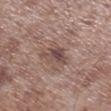Clinical impression:
The lesion was tiled from a total-body skin photograph and was not biopsied.
Context:
A 15 mm close-up tile from a total-body photography series done for melanoma screening. Imaged with white-light lighting. From the right lower leg. Longest diameter approximately 3.5 mm. A male subject, aged 53–57. An algorithmic analysis of the crop reported a footprint of about 5.5 mm², an eccentricity of roughly 0.75, and a symmetry-axis asymmetry near 0.4. It also reported a mean CIELAB color near L≈46 a*≈17 b*≈20 and a normalized border contrast of about 7.5. The software also gave internal color variation of about 4.5 on a 0–10 scale and radial color variation of about 1.5. The software also gave a nevus-likeness score of about 5/100 and lesion-presence confidence of about 100/100.